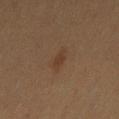Recorded during total-body skin imaging; not selected for excision or biopsy. The lesion is located on the left thigh. The patient is a female roughly 55 years of age. A region of skin cropped from a whole-body photographic capture, roughly 15 mm wide. Captured under cross-polarized illumination. The total-body-photography lesion software estimated a border-irregularity index near 2.5/10 and a peripheral color-asymmetry measure near 0. It also reported a nevus-likeness score of about 50/100 and a lesion-detection confidence of about 100/100. About 2.5 mm across.